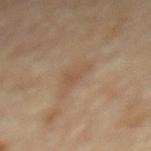Case summary:
• follow-up: total-body-photography surveillance lesion; no biopsy
• image: total-body-photography crop, ~15 mm field of view
• lighting: cross-polarized illumination
• site: the back
• patient: male, aged approximately 85 or older
• automated lesion analysis: a footprint of about 3.5 mm², an outline eccentricity of about 0.85 (0 = round, 1 = elongated), and two-axis asymmetry of about 0.5; a mean CIELAB color near L≈49 a*≈17 b*≈29, roughly 6 lightness units darker than nearby skin, and a normalized lesion–skin contrast near 5